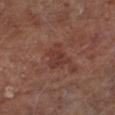Case summary:
- follow-up: catalogued during a skin exam; not biopsied
- anatomic site: the left lower leg
- image: 15 mm crop, total-body photography
- subject: male, roughly 65 years of age
- illumination: cross-polarized illumination